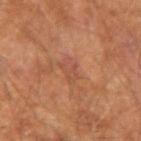Assessment:
This lesion was catalogued during total-body skin photography and was not selected for biopsy.
Background:
Captured under cross-polarized illumination. The subject is a male aged 63–67. On the left forearm. A lesion tile, about 15 mm wide, cut from a 3D total-body photograph. Measured at roughly 3 mm in maximum diameter.